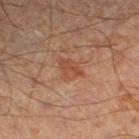Captured during whole-body skin photography for melanoma surveillance; the lesion was not biopsied.
The patient is a male aged 63 to 67.
Longest diameter approximately 3.5 mm.
The lesion is located on the left lower leg.
A 15 mm close-up extracted from a 3D total-body photography capture.